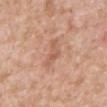No biopsy was performed on this lesion — it was imaged during a full skin examination and was not determined to be concerning. The tile uses white-light illumination. A male subject, about 60 years old. Located on the back. The lesion-visualizer software estimated a footprint of about 5 mm², a shape eccentricity near 0.85, and two-axis asymmetry of about 0.55. And it measured an average lesion color of about L≈59 a*≈23 b*≈31 (CIELAB), a lesion–skin lightness drop of about 8, and a normalized border contrast of about 5. The software also gave border irregularity of about 5.5 on a 0–10 scale, a color-variation rating of about 2.5/10, and peripheral color asymmetry of about 1. It also reported a classifier nevus-likeness of about 0/100 and a detector confidence of about 100 out of 100 that the crop contains a lesion. Approximately 3.5 mm at its widest. A 15 mm close-up extracted from a 3D total-body photography capture.